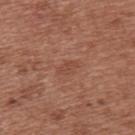<record>
  <biopsy_status>not biopsied; imaged during a skin examination</biopsy_status>
  <patient>
    <sex>female</sex>
    <age_approx>40</age_approx>
  </patient>
  <site>upper back</site>
  <image>
    <source>total-body photography crop</source>
    <field_of_view_mm>15</field_of_view_mm>
  </image>
</record>